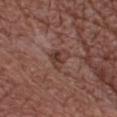The lesion was tiled from a total-body skin photograph and was not biopsied. This is a white-light tile. This image is a 15 mm lesion crop taken from a total-body photograph. From the chest. A male subject in their mid-70s. The lesion's longest dimension is about 3 mm.